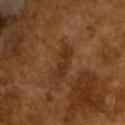Part of a total-body skin-imaging series; this lesion was reviewed on a skin check and was not flagged for biopsy. A male subject, aged 63–67. The recorded lesion diameter is about 4.5 mm. Automated image analysis of the tile measured a border-irregularity rating of about 4.5/10, internal color variation of about 1.5 on a 0–10 scale, and a peripheral color-asymmetry measure near 0.5. Cropped from a total-body skin-imaging series; the visible field is about 15 mm.